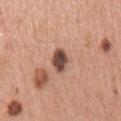Assessment: Part of a total-body skin-imaging series; this lesion was reviewed on a skin check and was not flagged for biopsy. Context: An algorithmic analysis of the crop reported a footprint of about 6 mm², an eccentricity of roughly 0.7, and a symmetry-axis asymmetry near 0.15. It also reported an average lesion color of about L≈49 a*≈20 b*≈25 (CIELAB) and a lesion–skin lightness drop of about 17. And it measured internal color variation of about 5 on a 0–10 scale and radial color variation of about 1.5. The lesion is located on the right upper arm. A 15 mm crop from a total-body photograph taken for skin-cancer surveillance. The tile uses white-light illumination. A male subject aged around 55.